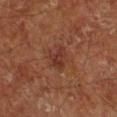  biopsy_status: not biopsied; imaged during a skin examination
  patient:
    sex: male
    age_approx: 65
  image:
    source: total-body photography crop
    field_of_view_mm: 15
  site: right lower leg
  lesion_size:
    long_diameter_mm_approx: 3.0